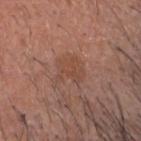Part of a total-body skin-imaging series; this lesion was reviewed on a skin check and was not flagged for biopsy. A region of skin cropped from a whole-body photographic capture, roughly 15 mm wide. Approximately 3.5 mm at its widest. A male subject aged around 45. The tile uses white-light illumination. The lesion is located on the head or neck.